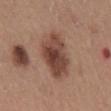The lesion was photographed on a routine skin check and not biopsied; there is no pathology result.
Longest diameter approximately 6 mm.
The lesion is on the back.
A male subject, in their mid-50s.
The total-body-photography lesion software estimated an area of roughly 17 mm², an outline eccentricity of about 0.75 (0 = round, 1 = elongated), and a shape-asymmetry score of about 0.2 (0 = symmetric). And it measured a mean CIELAB color near L≈44 a*≈21 b*≈25, roughly 13 lightness units darker than nearby skin, and a normalized border contrast of about 10. And it measured a border-irregularity rating of about 2.5/10, internal color variation of about 5.5 on a 0–10 scale, and peripheral color asymmetry of about 1.5.
Imaged with white-light lighting.
A roughly 15 mm field-of-view crop from a total-body skin photograph.